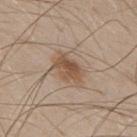Q: Was a biopsy performed?
A: catalogued during a skin exam; not biopsied
Q: Illumination type?
A: white-light illumination
Q: What are the patient's age and sex?
A: male, in their 30s
Q: What kind of image is this?
A: ~15 mm crop, total-body skin-cancer survey
Q: Automated lesion metrics?
A: an area of roughly 7.5 mm², a shape eccentricity near 0.8, and two-axis asymmetry of about 0.25; an average lesion color of about L≈52 a*≈16 b*≈30 (CIELAB), a lesion–skin lightness drop of about 10, and a lesion-to-skin contrast of about 8 (normalized; higher = more distinct); border irregularity of about 3 on a 0–10 scale, a color-variation rating of about 4/10, and peripheral color asymmetry of about 1.5
Q: What is the anatomic site?
A: the upper back